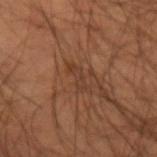  biopsy_status: not biopsied; imaged during a skin examination
  lesion_size:
    long_diameter_mm_approx: 3.5
  site: left forearm
  image:
    source: total-body photography crop
    field_of_view_mm: 15
  automated_metrics:
    vs_skin_contrast_norm: 5.0
    border_irregularity_0_10: 4.5
    color_variation_0_10: 0.0
  patient:
    sex: male
    age_approx: 55